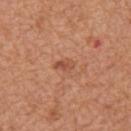The lesion was tiled from a total-body skin photograph and was not biopsied.
A male subject aged 63 to 67.
The lesion is located on the mid back.
A close-up tile cropped from a whole-body skin photograph, about 15 mm across.